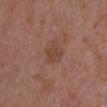Q: Was a biopsy performed?
A: no biopsy performed (imaged during a skin exam)
Q: What kind of image is this?
A: 15 mm crop, total-body photography
Q: What are the patient's age and sex?
A: female, in their mid-30s
Q: What is the anatomic site?
A: the chest